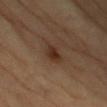Imaged during a routine full-body skin examination; the lesion was not biopsied and no histopathology is available. On the right upper arm. The lesion's longest dimension is about 3 mm. The tile uses cross-polarized illumination. A female subject about 70 years old. This image is a 15 mm lesion crop taken from a total-body photograph.